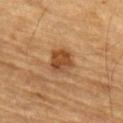Captured during whole-body skin photography for melanoma surveillance; the lesion was not biopsied.
The lesion is on the arm.
The subject is a male approximately 85 years of age or older.
The recorded lesion diameter is about 3.5 mm.
A lesion tile, about 15 mm wide, cut from a 3D total-body photograph.